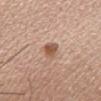<tbp_lesion>
  <biopsy_status>not biopsied; imaged during a skin examination</biopsy_status>
  <patient>
    <sex>female</sex>
    <age_approx>55</age_approx>
  </patient>
  <image>
    <source>total-body photography crop</source>
    <field_of_view_mm>15</field_of_view_mm>
  </image>
  <site>head or neck</site>
  <lighting>white-light</lighting>
</tbp_lesion>